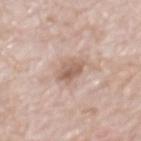Case summary:
• follow-up: imaged on a skin check; not biopsied
• site: the mid back
• subject: male, approximately 70 years of age
• imaging modality: ~15 mm crop, total-body skin-cancer survey